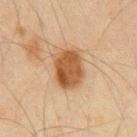Impression:
Part of a total-body skin-imaging series; this lesion was reviewed on a skin check and was not flagged for biopsy.
Context:
The recorded lesion diameter is about 5 mm. This is a cross-polarized tile. The total-body-photography lesion software estimated a lesion area of about 15 mm², an outline eccentricity of about 0.7 (0 = round, 1 = elongated), and a symmetry-axis asymmetry near 0.15. The software also gave an average lesion color of about L≈45 a*≈18 b*≈32 (CIELAB), a lesion–skin lightness drop of about 12, and a normalized border contrast of about 9.5. It also reported a border-irregularity rating of about 1.5/10, a within-lesion color-variation index near 4.5/10, and a peripheral color-asymmetry measure near 1.5. The analysis additionally found a classifier nevus-likeness of about 100/100. The patient is a male in their mid-60s. A 15 mm close-up tile from a total-body photography series done for melanoma screening. The lesion is on the chest.